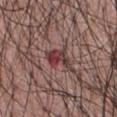Clinical impression: Imaged during a routine full-body skin examination; the lesion was not biopsied and no histopathology is available.